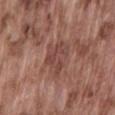  biopsy_status: not biopsied; imaged during a skin examination
  patient:
    sex: male
    age_approx: 75
  image:
    source: total-body photography crop
    field_of_view_mm: 15
  site: lower back